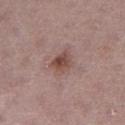Q: Was this lesion biopsied?
A: total-body-photography surveillance lesion; no biopsy
Q: Patient demographics?
A: female, roughly 40 years of age
Q: Automated lesion metrics?
A: a classifier nevus-likeness of about 75/100 and a lesion-detection confidence of about 100/100
Q: How was this image acquired?
A: total-body-photography crop, ~15 mm field of view
Q: What is the lesion's diameter?
A: ~3 mm (longest diameter)
Q: Lesion location?
A: the left thigh
Q: What lighting was used for the tile?
A: white-light illumination